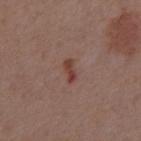The lesion was tiled from a total-body skin photograph and was not biopsied. Longest diameter approximately 2.5 mm. Cropped from a total-body skin-imaging series; the visible field is about 15 mm. Automated image analysis of the tile measured a mean CIELAB color near L≈40 a*≈23 b*≈24 and a lesion-to-skin contrast of about 8 (normalized; higher = more distinct). And it measured an automated nevus-likeness rating near 10 out of 100 and a lesion-detection confidence of about 100/100. A male patient, roughly 55 years of age. The lesion is on the chest. This is a white-light tile.